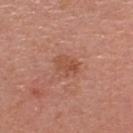Captured during whole-body skin photography for melanoma surveillance; the lesion was not biopsied. Captured under white-light illumination. Located on the head or neck. Cropped from a total-body skin-imaging series; the visible field is about 15 mm. About 3 mm across. A female subject aged approximately 50.